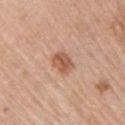workup — imaged on a skin check; not biopsied
patient — female, aged 63–67
image source — 15 mm crop, total-body photography
body site — the right upper arm